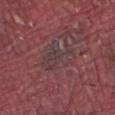Clinical impression: This lesion was catalogued during total-body skin photography and was not selected for biopsy. Image and clinical context: Imaged with white-light lighting. Cropped from a whole-body photographic skin survey; the tile spans about 15 mm. On the right forearm. The lesion-visualizer software estimated a lesion color around L≈37 a*≈18 b*≈14 in CIELAB, a lesion–skin lightness drop of about 4, and a normalized border contrast of about 5. The software also gave an automated nevus-likeness rating near 0 out of 100. A male subject, roughly 40 years of age.